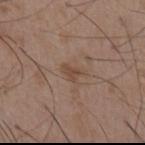The patient is a male aged around 55.
The tile uses white-light illumination.
The lesion is on the abdomen.
Automated image analysis of the tile measured border irregularity of about 4.5 on a 0–10 scale, a within-lesion color-variation index near 1/10, and a peripheral color-asymmetry measure near 0.5. The analysis additionally found a classifier nevus-likeness of about 0/100.
The lesion's longest dimension is about 2.5 mm.
A 15 mm close-up extracted from a 3D total-body photography capture.